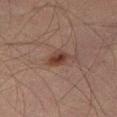Clinical impression:
The lesion was photographed on a routine skin check and not biopsied; there is no pathology result.
Background:
Automated tile analysis of the lesion measured a shape eccentricity near 0.65 and a shape-asymmetry score of about 0.25 (0 = symmetric). The analysis additionally found radial color variation of about 1. This image is a 15 mm lesion crop taken from a total-body photograph. Measured at roughly 2.5 mm in maximum diameter. A male subject about 30 years old. On the right thigh.